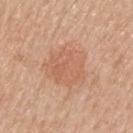{"biopsy_status": "not biopsied; imaged during a skin examination", "lighting": "white-light", "patient": {"sex": "male", "age_approx": 60}, "automated_metrics": {"cielab_L": 61, "cielab_a": 22, "cielab_b": 33, "vs_skin_darker_L": 7.0, "border_irregularity_0_10": 3.5, "color_variation_0_10": 3.0, "peripheral_color_asymmetry": 1.0}, "lesion_size": {"long_diameter_mm_approx": 5.5}, "image": {"source": "total-body photography crop", "field_of_view_mm": 15}, "site": "back"}